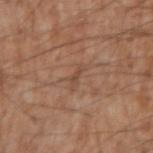Case summary:
- biopsy status · catalogued during a skin exam; not biopsied
- automated metrics · a footprint of about 2.5 mm², an eccentricity of roughly 0.9, and two-axis asymmetry of about 0.4; a border-irregularity rating of about 4.5/10, a color-variation rating of about 0/10, and peripheral color asymmetry of about 0; an automated nevus-likeness rating near 0 out of 100 and lesion-presence confidence of about 90/100
- image · ~15 mm tile from a whole-body skin photo
- patient · male, roughly 75 years of age
- tile lighting · white-light illumination
- site · the right upper arm
- lesion diameter · about 3 mm